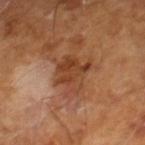{"biopsy_status": "not biopsied; imaged during a skin examination", "image": {"source": "total-body photography crop", "field_of_view_mm": 15}, "patient": {"sex": "male", "age_approx": 65}}